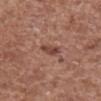notes: total-body-photography surveillance lesion; no biopsy